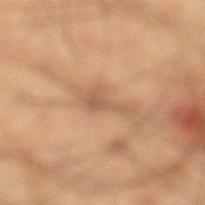Recorded during total-body skin imaging; not selected for excision or biopsy. Cropped from a total-body skin-imaging series; the visible field is about 15 mm. Measured at roughly 4 mm in maximum diameter. Captured under cross-polarized illumination. On the left lower leg. A male patient, aged approximately 40.